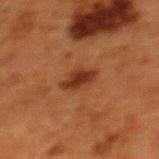biopsy status: no biopsy performed (imaged during a skin exam); site: the upper back; image source: total-body-photography crop, ~15 mm field of view; subject: female, roughly 50 years of age.